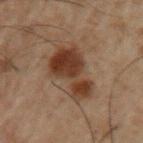No biopsy was performed on this lesion — it was imaged during a full skin examination and was not determined to be concerning.
A 15 mm close-up extracted from a 3D total-body photography capture.
The lesion's longest dimension is about 6.5 mm.
A male subject, aged approximately 50.
From the arm.